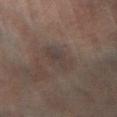No biopsy was performed on this lesion — it was imaged during a full skin examination and was not determined to be concerning. Cropped from a total-body skin-imaging series; the visible field is about 15 mm. A male patient aged approximately 65. From the left lower leg.